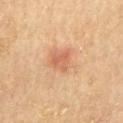biopsy status: imaged on a skin check; not biopsied
image-analysis metrics: a lesion color around L≈64 a*≈21 b*≈35 in CIELAB and about 7 CIELAB-L* units darker than the surrounding skin
lesion size: ≈4.5 mm
tile lighting: cross-polarized illumination
patient: male, roughly 85 years of age or older
body site: the chest
image source: 15 mm crop, total-body photography